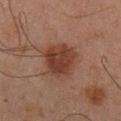Findings:
– biopsy status · catalogued during a skin exam; not biopsied
– acquisition · total-body-photography crop, ~15 mm field of view
– location · the leg
– subject · male, about 65 years old